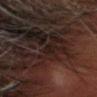Assessment: The lesion was photographed on a routine skin check and not biopsied; there is no pathology result. Acquisition and patient details: The lesion-visualizer software estimated a mean CIELAB color near L≈17 a*≈12 b*≈16 and a lesion-to-skin contrast of about 5 (normalized; higher = more distinct). The analysis additionally found a border-irregularity rating of about 2/10, internal color variation of about 0 on a 0–10 scale, and a peripheral color-asymmetry measure near 0. And it measured an automated nevus-likeness rating near 0 out of 100. Captured under cross-polarized illumination. Located on the head or neck. A 15 mm close-up tile from a total-body photography series done for melanoma screening. A male subject, aged 53 to 57. Approximately 1.5 mm at its widest.